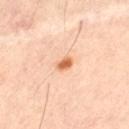<case>
<biopsy_status>not biopsied; imaged during a skin examination</biopsy_status>
<site>leg</site>
<image>
  <source>total-body photography crop</source>
  <field_of_view_mm>15</field_of_view_mm>
</image>
<lighting>cross-polarized</lighting>
<patient>
  <sex>male</sex>
  <age_approx>65</age_approx>
</patient>
<lesion_size>
  <long_diameter_mm_approx>2.0</long_diameter_mm_approx>
</lesion_size>
</case>